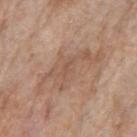workup — catalogued during a skin exam; not biopsied
anatomic site — the arm
lighting — white-light illumination
subject — female, approximately 85 years of age or older
TBP lesion metrics — a lesion area of about 15 mm², an outline eccentricity of about 0.95 (0 = round, 1 = elongated), and a shape-asymmetry score of about 0.55 (0 = symmetric); a mean CIELAB color near L≈55 a*≈18 b*≈28, about 8 CIELAB-L* units darker than the surrounding skin, and a lesion-to-skin contrast of about 5.5 (normalized; higher = more distinct); peripheral color asymmetry of about 0.5; a nevus-likeness score of about 0/100 and lesion-presence confidence of about 55/100
image — total-body-photography crop, ~15 mm field of view
lesion size — ≈8.5 mm